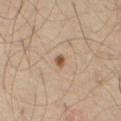A male patient, about 65 years old.
The recorded lesion diameter is about 1.5 mm.
Imaged with cross-polarized lighting.
Located on the abdomen.
A close-up tile cropped from a whole-body skin photograph, about 15 mm across.